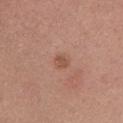notes: imaged on a skin check; not biopsied | image-analysis metrics: a lesion–skin lightness drop of about 8; border irregularity of about 2 on a 0–10 scale, a within-lesion color-variation index near 1/10, and peripheral color asymmetry of about 0.5; a nevus-likeness score of about 30/100 and a lesion-detection confidence of about 100/100 | acquisition: ~15 mm tile from a whole-body skin photo | body site: the head or neck | patient: female, aged 33 to 37.